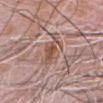{
  "biopsy_status": "not biopsied; imaged during a skin examination",
  "site": "head or neck",
  "patient": {
    "sex": "male",
    "age_approx": 80
  },
  "lesion_size": {
    "long_diameter_mm_approx": 3.5
  },
  "image": {
    "source": "total-body photography crop",
    "field_of_view_mm": 15
  },
  "automated_metrics": {
    "area_mm2_approx": 6.0,
    "eccentricity": 0.85,
    "shape_asymmetry": 0.35,
    "cielab_L": 52,
    "cielab_a": 20,
    "cielab_b": 27,
    "vs_skin_darker_L": 9.0,
    "vs_skin_contrast_norm": 7.5,
    "nevus_likeness_0_100": 0
  },
  "lighting": "white-light"
}